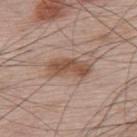image:
  source: total-body photography crop
  field_of_view_mm: 15
patient:
  sex: male
  age_approx: 65
site: upper back
lesion_size:
  long_diameter_mm_approx: 5.0
lighting: white-light